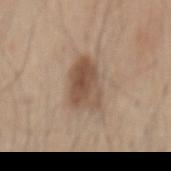Approximately 5 mm at its widest. A male subject aged approximately 45. A lesion tile, about 15 mm wide, cut from a 3D total-body photograph. On the mid back.